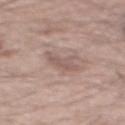The lesion was photographed on a routine skin check and not biopsied; there is no pathology result.
The tile uses white-light illumination.
Approximately 3.5 mm at its widest.
A 15 mm close-up extracted from a 3D total-body photography capture.
Automated tile analysis of the lesion measured a border-irregularity index near 5/10 and peripheral color asymmetry of about 1.
The lesion is located on the mid back.
The subject is a male aged around 55.